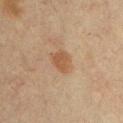| key | value |
|---|---|
| patient | male, aged approximately 60 |
| image-analysis metrics | a footprint of about 6 mm²; an average lesion color of about L≈44 a*≈16 b*≈29 (CIELAB), about 7 CIELAB-L* units darker than the surrounding skin, and a normalized border contrast of about 6.5; border irregularity of about 2 on a 0–10 scale, internal color variation of about 2 on a 0–10 scale, and a peripheral color-asymmetry measure near 0.5; a nevus-likeness score of about 80/100 |
| image | ~15 mm tile from a whole-body skin photo |
| tile lighting | cross-polarized |
| size | ~3 mm (longest diameter) |
| location | the chest |